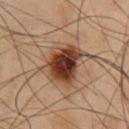No biopsy was performed on this lesion — it was imaged during a full skin examination and was not determined to be concerning. A 15 mm close-up extracted from a 3D total-body photography capture. Captured under cross-polarized illumination. Automated image analysis of the tile measured an eccentricity of roughly 0.75 and a symmetry-axis asymmetry near 0.25. And it measured a mean CIELAB color near L≈26 a*≈19 b*≈24. It also reported a border-irregularity rating of about 2.5/10, internal color variation of about 5.5 on a 0–10 scale, and radial color variation of about 1.5. A male patient, aged 53–57. On the chest.